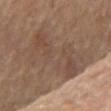Clinical impression: No biopsy was performed on this lesion — it was imaged during a full skin examination and was not determined to be concerning. Context: Captured under cross-polarized illumination. Measured at roughly 7.5 mm in maximum diameter. The subject is a male about 70 years old. This image is a 15 mm lesion crop taken from a total-body photograph. From the chest. Automated image analysis of the tile measured an area of roughly 31 mm², a shape eccentricity near 0.75, and a symmetry-axis asymmetry near 0.35. The analysis additionally found a mean CIELAB color near L≈45 a*≈15 b*≈24 and a lesion–skin lightness drop of about 6. And it measured a classifier nevus-likeness of about 0/100 and a detector confidence of about 65 out of 100 that the crop contains a lesion.